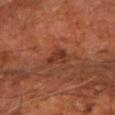No biopsy was performed on this lesion — it was imaged during a full skin examination and was not determined to be concerning. Imaged with cross-polarized lighting. The total-body-photography lesion software estimated an eccentricity of roughly 0.55 and a symmetry-axis asymmetry near 0.4. The analysis additionally found a border-irregularity index near 4/10, a within-lesion color-variation index near 2/10, and radial color variation of about 0.5. The analysis additionally found a lesion-detection confidence of about 100/100. Longest diameter approximately 2.5 mm. From the right forearm. A region of skin cropped from a whole-body photographic capture, roughly 15 mm wide. The patient is a male aged around 60.